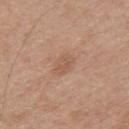Clinical impression: Recorded during total-body skin imaging; not selected for excision or biopsy. Context: From the upper back. Cropped from a whole-body photographic skin survey; the tile spans about 15 mm. The tile uses white-light illumination. A male patient in their 30s.